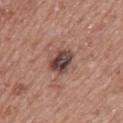{
  "biopsy_status": "not biopsied; imaged during a skin examination",
  "automated_metrics": {
    "area_mm2_approx": 8.0,
    "eccentricity": 0.6,
    "shape_asymmetry": 0.15,
    "cielab_L": 44,
    "cielab_a": 19,
    "cielab_b": 22,
    "vs_skin_darker_L": 14.0,
    "lesion_detection_confidence_0_100": 100
  },
  "patient": {
    "sex": "female",
    "age_approx": 60
  },
  "lesion_size": {
    "long_diameter_mm_approx": 3.5
  },
  "lighting": "white-light",
  "image": {
    "source": "total-body photography crop",
    "field_of_view_mm": 15
  },
  "site": "upper back"
}